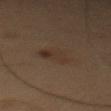Findings:
- workup · catalogued during a skin exam; not biopsied
- location · the mid back
- TBP lesion metrics · an outline eccentricity of about 0.7 (0 = round, 1 = elongated); roughly 4 lightness units darker than nearby skin and a normalized border contrast of about 5; a detector confidence of about 100 out of 100 that the crop contains a lesion
- diameter · about 4 mm
- lighting · cross-polarized
- image · total-body-photography crop, ~15 mm field of view
- subject · male, aged approximately 55